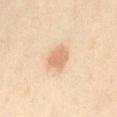  biopsy_status: not biopsied; imaged during a skin examination
  patient:
    sex: female
    age_approx: 40
  site: abdomen
  image:
    source: total-body photography crop
    field_of_view_mm: 15
  lesion_size:
    long_diameter_mm_approx: 3.0
  lighting: cross-polarized